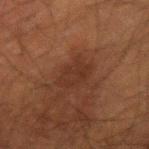Clinical impression: This lesion was catalogued during total-body skin photography and was not selected for biopsy. Acquisition and patient details: The subject is a male aged around 50. A 15 mm close-up tile from a total-body photography series done for melanoma screening. Located on the right upper arm.